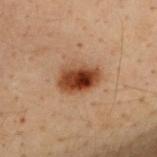Captured during whole-body skin photography for melanoma surveillance; the lesion was not biopsied.
Captured under cross-polarized illumination.
A roughly 15 mm field-of-view crop from a total-body skin photograph.
Located on the upper back.
Automated image analysis of the tile measured lesion-presence confidence of about 100/100.
About 4.5 mm across.
The patient is a male aged 28 to 32.